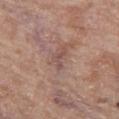Impression:
The lesion was tiled from a total-body skin photograph and was not biopsied.
Acquisition and patient details:
Automated image analysis of the tile measured an area of roughly 2.5 mm², an outline eccentricity of about 0.8 (0 = round, 1 = elongated), and a symmetry-axis asymmetry near 0.7. The analysis additionally found a lesion color around L≈50 a*≈19 b*≈23 in CIELAB and about 7 CIELAB-L* units darker than the surrounding skin. It also reported a border-irregularity rating of about 7.5/10 and peripheral color asymmetry of about 0. A region of skin cropped from a whole-body photographic capture, roughly 15 mm wide. The lesion is located on the leg. The recorded lesion diameter is about 2.5 mm. The subject is a female roughly 75 years of age.